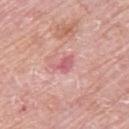A 15 mm close-up tile from a total-body photography series done for melanoma screening.
From the upper back.
The patient is a female approximately 65 years of age.